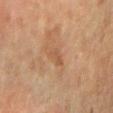The lesion was photographed on a routine skin check and not biopsied; there is no pathology result. A lesion tile, about 15 mm wide, cut from a 3D total-body photograph. Automated image analysis of the tile measured an area of roughly 2.5 mm², an eccentricity of roughly 0.9, and a symmetry-axis asymmetry near 0.35. The analysis additionally found a border-irregularity index near 3.5/10 and radial color variation of about 0. A female subject aged around 55. The lesion is located on the right forearm. Imaged with cross-polarized lighting.